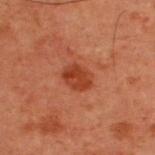Case summary:
– notes — total-body-photography surveillance lesion; no biopsy
– subject — male, about 60 years old
– imaging modality — ~15 mm crop, total-body skin-cancer survey
– lesion diameter — ≈3.5 mm
– site — the upper back